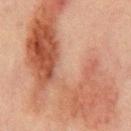Assessment: Recorded during total-body skin imaging; not selected for excision or biopsy. Acquisition and patient details: The tile uses cross-polarized illumination. From the mid back. The subject is a male aged 63–67. An algorithmic analysis of the crop reported an area of roughly 85 mm². The analysis additionally found a color-variation rating of about 7.5/10 and a peripheral color-asymmetry measure near 2.5. A region of skin cropped from a whole-body photographic capture, roughly 15 mm wide. About 17 mm across.